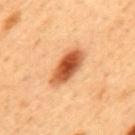Assessment:
This lesion was catalogued during total-body skin photography and was not selected for biopsy.
Clinical summary:
The lesion is located on the mid back. The lesion's longest dimension is about 5 mm. Cropped from a whole-body photographic skin survey; the tile spans about 15 mm. The tile uses cross-polarized illumination. A male subject aged approximately 50.